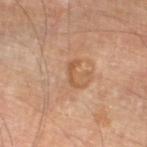biopsy status: no biopsy performed (imaged during a skin exam) | size: about 3 mm | body site: the left lower leg | patient: male, approximately 65 years of age | lighting: cross-polarized illumination | image source: ~15 mm tile from a whole-body skin photo | TBP lesion metrics: an average lesion color of about L≈52 a*≈20 b*≈34 (CIELAB), about 8 CIELAB-L* units darker than the surrounding skin, and a lesion-to-skin contrast of about 6 (normalized; higher = more distinct).